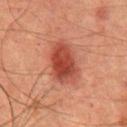Clinical impression: Part of a total-body skin-imaging series; this lesion was reviewed on a skin check and was not flagged for biopsy. Acquisition and patient details: Located on the chest. A roughly 15 mm field-of-view crop from a total-body skin photograph. Imaged with cross-polarized lighting. The recorded lesion diameter is about 5.5 mm. A male subject, approximately 65 years of age. Automated image analysis of the tile measured a mean CIELAB color near L≈43 a*≈29 b*≈30, about 12 CIELAB-L* units darker than the surrounding skin, and a lesion-to-skin contrast of about 9 (normalized; higher = more distinct). The software also gave border irregularity of about 2 on a 0–10 scale, internal color variation of about 5 on a 0–10 scale, and a peripheral color-asymmetry measure near 1.5.